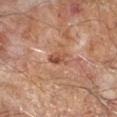notes = no biopsy performed (imaged during a skin exam); image source = ~15 mm tile from a whole-body skin photo; patient = male, aged approximately 65; tile lighting = cross-polarized; automated lesion analysis = a lesion color around L≈49 a*≈23 b*≈31 in CIELAB, about 9 CIELAB-L* units darker than the surrounding skin, and a normalized lesion–skin contrast near 6.5; size = about 2.5 mm; location = the right forearm.